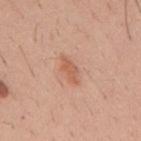workup — total-body-photography surveillance lesion; no biopsy | imaging modality — ~15 mm crop, total-body skin-cancer survey | body site — the mid back | subject — male, roughly 30 years of age.